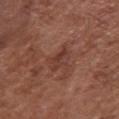Part of a total-body skin-imaging series; this lesion was reviewed on a skin check and was not flagged for biopsy.
The subject is a female about 75 years old.
The lesion is on the front of the torso.
A 15 mm close-up extracted from a 3D total-body photography capture.
Approximately 2.5 mm at its widest.